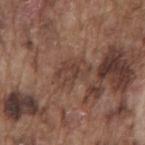Q: Is there a histopathology result?
A: imaged on a skin check; not biopsied
Q: How was this image acquired?
A: 15 mm crop, total-body photography
Q: What did automated image analysis measure?
A: border irregularity of about 3 on a 0–10 scale, internal color variation of about 3 on a 0–10 scale, and a peripheral color-asymmetry measure near 1; a lesion-detection confidence of about 60/100
Q: Where on the body is the lesion?
A: the back
Q: How was the tile lit?
A: white-light
Q: Patient demographics?
A: male, in their mid-70s